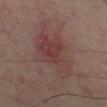Captured during whole-body skin photography for melanoma surveillance; the lesion was not biopsied. A male patient in their mid- to late 70s. From the right lower leg. A close-up tile cropped from a whole-body skin photograph, about 15 mm across. The total-body-photography lesion software estimated a border-irregularity index near 5/10, a color-variation rating of about 4.5/10, and radial color variation of about 1.5. Longest diameter approximately 6.5 mm.